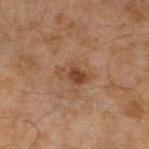No biopsy was performed on this lesion — it was imaged during a full skin examination and was not determined to be concerning.
This is a cross-polarized tile.
The lesion is on the leg.
A close-up tile cropped from a whole-body skin photograph, about 15 mm across.
An algorithmic analysis of the crop reported a lesion area of about 5.5 mm² and a shape-asymmetry score of about 0.25 (0 = symmetric). It also reported an average lesion color of about L≈35 a*≈18 b*≈27 (CIELAB), roughly 8 lightness units darker than nearby skin, and a normalized border contrast of about 7.5. It also reported a nevus-likeness score of about 60/100.
The recorded lesion diameter is about 3.5 mm.
A male patient in their mid-40s.